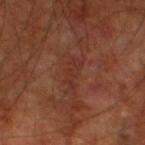Impression:
Imaged during a routine full-body skin examination; the lesion was not biopsied and no histopathology is available.
Background:
The subject is a male roughly 60 years of age. Measured at roughly 3.5 mm in maximum diameter. Automated tile analysis of the lesion measured an automated nevus-likeness rating near 0 out of 100 and a detector confidence of about 70 out of 100 that the crop contains a lesion. A 15 mm close-up tile from a total-body photography series done for melanoma screening. From the left thigh. Captured under cross-polarized illumination.